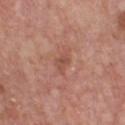  biopsy_status: not biopsied; imaged during a skin examination
  lighting: white-light
  patient:
    sex: male
    age_approx: 65
  image:
    source: total-body photography crop
    field_of_view_mm: 15
  lesion_size:
    long_diameter_mm_approx: 2.5
  site: chest